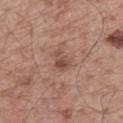Captured during whole-body skin photography for melanoma surveillance; the lesion was not biopsied.
The recorded lesion diameter is about 2.5 mm.
On the back.
The tile uses white-light illumination.
A male subject roughly 55 years of age.
A region of skin cropped from a whole-body photographic capture, roughly 15 mm wide.